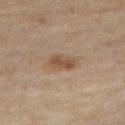site: the right thigh
patient: female, aged around 50
imaging modality: ~15 mm crop, total-body skin-cancer survey